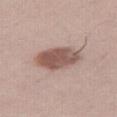<lesion>
  <biopsy_status>not biopsied; imaged during a skin examination</biopsy_status>
  <automated_metrics>
    <border_irregularity_0_10>1.5</border_irregularity_0_10>
    <color_variation_0_10>4.0</color_variation_0_10>
    <peripheral_color_asymmetry>1.5</peripheral_color_asymmetry>
  </automated_metrics>
  <image>
    <source>total-body photography crop</source>
    <field_of_view_mm>15</field_of_view_mm>
  </image>
  <patient>
    <sex>female</sex>
    <age_approx>40</age_approx>
  </patient>
  <site>right thigh</site>
  <lighting>white-light</lighting>
  <lesion_size>
    <long_diameter_mm_approx>5.5</long_diameter_mm_approx>
  </lesion_size>
</lesion>